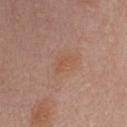Impression:
Part of a total-body skin-imaging series; this lesion was reviewed on a skin check and was not flagged for biopsy.
Acquisition and patient details:
The lesion is located on the chest. The subject is a male roughly 30 years of age. A 15 mm close-up tile from a total-body photography series done for melanoma screening. The tile uses white-light illumination. The total-body-photography lesion software estimated a footprint of about 2.5 mm², an eccentricity of roughly 0.85, and a shape-asymmetry score of about 0.5 (0 = symmetric). The software also gave a mean CIELAB color near L≈53 a*≈21 b*≈31, about 5 CIELAB-L* units darker than the surrounding skin, and a normalized lesion–skin contrast near 4.5. The software also gave an automated nevus-likeness rating near 20 out of 100.